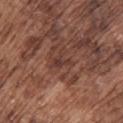• workup — no biopsy performed (imaged during a skin exam)
• patient — male, aged approximately 75
• body site — the upper back
• image source — ~15 mm crop, total-body skin-cancer survey